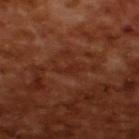Q: Is there a histopathology result?
A: imaged on a skin check; not biopsied
Q: What lighting was used for the tile?
A: cross-polarized
Q: What is the imaging modality?
A: total-body-photography crop, ~15 mm field of view
Q: What are the patient's age and sex?
A: male, aged 63–67
Q: Automated lesion metrics?
A: an area of roughly 3 mm², an eccentricity of roughly 0.9, and a shape-asymmetry score of about 0.45 (0 = symmetric); a nevus-likeness score of about 0/100 and a detector confidence of about 65 out of 100 that the crop contains a lesion